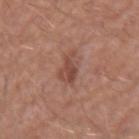Findings:
- patient · male, in their mid- to late 50s
- acquisition · 15 mm crop, total-body photography
- site · the right forearm